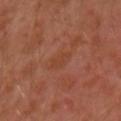<tbp_lesion>
  <biopsy_status>not biopsied; imaged during a skin examination</biopsy_status>
</tbp_lesion>